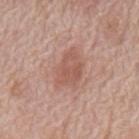The lesion was photographed on a routine skin check and not biopsied; there is no pathology result. On the mid back. Automated image analysis of the tile measured a footprint of about 9.5 mm², an outline eccentricity of about 0.75 (0 = round, 1 = elongated), and two-axis asymmetry of about 0.35. The software also gave a lesion color around L≈55 a*≈23 b*≈27 in CIELAB, about 8 CIELAB-L* units darker than the surrounding skin, and a lesion-to-skin contrast of about 6 (normalized; higher = more distinct). And it measured a detector confidence of about 100 out of 100 that the crop contains a lesion. A male subject approximately 70 years of age. Cropped from a whole-body photographic skin survey; the tile spans about 15 mm. Captured under white-light illumination. Approximately 4.5 mm at its widest.